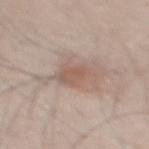Assessment: No biopsy was performed on this lesion — it was imaged during a full skin examination and was not determined to be concerning. Clinical summary: A 15 mm close-up tile from a total-body photography series done for melanoma screening. The patient is a male aged 33–37. Measured at roughly 3.5 mm in maximum diameter. The lesion is on the mid back. The lesion-visualizer software estimated a border-irregularity rating of about 3/10. The software also gave an automated nevus-likeness rating near 35 out of 100 and a detector confidence of about 100 out of 100 that the crop contains a lesion. The tile uses white-light illumination.